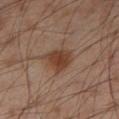biopsy_status: not biopsied; imaged during a skin examination
automated_metrics:
  color_variation_0_10: 3.5
  peripheral_color_asymmetry: 1.0
  nevus_likeness_0_100: 90
  lesion_detection_confidence_0_100: 100
lighting: cross-polarized
image:
  source: total-body photography crop
  field_of_view_mm: 15
patient:
  sex: male
  age_approx: 55
site: left lower leg